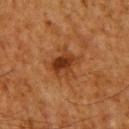Clinical impression: Imaged during a routine full-body skin examination; the lesion was not biopsied and no histopathology is available. Acquisition and patient details: A 15 mm close-up tile from a total-body photography series done for melanoma screening. Captured under cross-polarized illumination. A male subject, aged approximately 60. Approximately 4 mm at its widest. Located on the upper back. The total-body-photography lesion software estimated an average lesion color of about L≈33 a*≈21 b*≈32 (CIELAB), a lesion–skin lightness drop of about 8, and a lesion-to-skin contrast of about 7.5 (normalized; higher = more distinct).